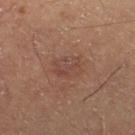Findings:
* follow-up: catalogued during a skin exam; not biopsied
* patient: male, roughly 60 years of age
* image-analysis metrics: an automated nevus-likeness rating near 10 out of 100
* image: ~15 mm crop, total-body skin-cancer survey
* location: the right thigh
* illumination: cross-polarized illumination